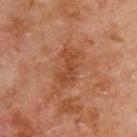Part of a total-body skin-imaging series; this lesion was reviewed on a skin check and was not flagged for biopsy.
A male patient aged approximately 70.
A close-up tile cropped from a whole-body skin photograph, about 15 mm across.
On the upper back.
Longest diameter approximately 4.5 mm.